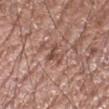Impression:
The lesion was tiled from a total-body skin photograph and was not biopsied.
Acquisition and patient details:
A male patient aged approximately 55. This is a white-light tile. A roughly 15 mm field-of-view crop from a total-body skin photograph. The lesion is located on the right forearm. The recorded lesion diameter is about 3 mm.